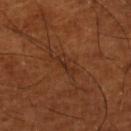The lesion was photographed on a routine skin check and not biopsied; there is no pathology result.
The tile uses cross-polarized illumination.
Longest diameter approximately 3 mm.
Automated image analysis of the tile measured an area of roughly 3 mm² and a shape-asymmetry score of about 0.45 (0 = symmetric). The software also gave roughly 6 lightness units darker than nearby skin and a lesion-to-skin contrast of about 6 (normalized; higher = more distinct). And it measured a border-irregularity index near 5/10 and a within-lesion color-variation index near 0/10. And it measured a classifier nevus-likeness of about 0/100 and a detector confidence of about 65 out of 100 that the crop contains a lesion.
Located on the right lower leg.
Cropped from a total-body skin-imaging series; the visible field is about 15 mm.
A male subject aged approximately 65.